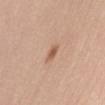<record>
<automated_metrics>
  <cielab_L>58</cielab_L>
  <cielab_a>21</cielab_a>
  <cielab_b>31</cielab_b>
  <vs_skin_darker_L>10.0</vs_skin_darker_L>
  <vs_skin_contrast_norm>7.0</vs_skin_contrast_norm>
</automated_metrics>
<patient>
  <sex>female</sex>
  <age_approx>40</age_approx>
</patient>
<lesion_size>
  <long_diameter_mm_approx>2.5</long_diameter_mm_approx>
</lesion_size>
<lighting>white-light</lighting>
<site>abdomen</site>
<image>
  <source>total-body photography crop</source>
  <field_of_view_mm>15</field_of_view_mm>
</image>
</record>